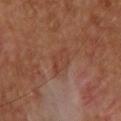follow-up: no biopsy performed (imaged during a skin exam)
diameter: ≈3 mm
location: the upper back
image: total-body-photography crop, ~15 mm field of view
illumination: cross-polarized illumination
patient: male, in their 60s